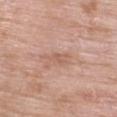Findings:
• follow-up · total-body-photography surveillance lesion; no biopsy
• image · ~15 mm tile from a whole-body skin photo
• subject · female, aged approximately 70
• illumination · white-light
• site · the upper back
• diameter · ≈2.5 mm